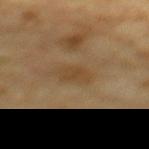Part of a total-body skin-imaging series; this lesion was reviewed on a skin check and was not flagged for biopsy.
A region of skin cropped from a whole-body photographic capture, roughly 15 mm wide.
Imaged with cross-polarized lighting.
A male subject approximately 70 years of age.
The lesion is on the mid back.